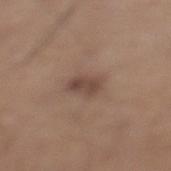Q: Was a biopsy performed?
A: total-body-photography surveillance lesion; no biopsy